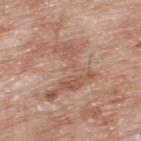A male patient roughly 60 years of age. A close-up tile cropped from a whole-body skin photograph, about 15 mm across. Located on the upper back. Longest diameter approximately 7.5 mm. The tile uses white-light illumination.